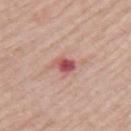Captured during whole-body skin photography for melanoma surveillance; the lesion was not biopsied. The lesion is on the left upper arm. Approximately 2.5 mm at its widest. The patient is a male aged 63 to 67. A close-up tile cropped from a whole-body skin photograph, about 15 mm across. The total-body-photography lesion software estimated a mean CIELAB color near L≈53 a*≈30 b*≈22, about 14 CIELAB-L* units darker than the surrounding skin, and a normalized border contrast of about 9.5. The software also gave internal color variation of about 5.5 on a 0–10 scale. It also reported an automated nevus-likeness rating near 0 out of 100 and lesion-presence confidence of about 100/100.